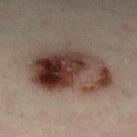Image and clinical context:
A lesion tile, about 15 mm wide, cut from a 3D total-body photograph. The recorded lesion diameter is about 9 mm. A male subject, aged 48 to 52. The lesion is located on the left leg. Imaged with cross-polarized lighting.